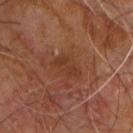Part of a total-body skin-imaging series; this lesion was reviewed on a skin check and was not flagged for biopsy.
The lesion is on the upper back.
Imaged with cross-polarized lighting.
An algorithmic analysis of the crop reported a nevus-likeness score of about 0/100.
Cropped from a total-body skin-imaging series; the visible field is about 15 mm.
A male subject aged around 60.